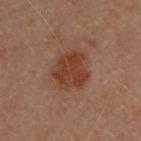follow-up: catalogued during a skin exam; not biopsied | illumination: cross-polarized illumination | diameter: about 4.5 mm | automated lesion analysis: an area of roughly 13 mm², an outline eccentricity of about 0.5 (0 = round, 1 = elongated), and two-axis asymmetry of about 0.25; an average lesion color of about L≈31 a*≈20 b*≈25 (CIELAB), a lesion–skin lightness drop of about 7, and a normalized border contrast of about 8; a nevus-likeness score of about 85/100 and a lesion-detection confidence of about 100/100 | subject: female, aged 28–32 | site: the upper back | acquisition: ~15 mm tile from a whole-body skin photo.